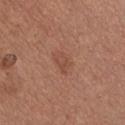notes: catalogued during a skin exam; not biopsied
imaging modality: 15 mm crop, total-body photography
diameter: about 2.5 mm
body site: the chest
patient: male, aged 33 to 37